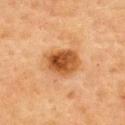biopsy_status: not biopsied; imaged during a skin examination
lesion_size:
  long_diameter_mm_approx: 4.5
automated_metrics:
  cielab_L: 47
  cielab_a: 24
  cielab_b: 39
  vs_skin_darker_L: 14.0
  nevus_likeness_0_100: 95
  lesion_detection_confidence_0_100: 100
site: upper back
image:
  source: total-body photography crop
  field_of_view_mm: 15
patient:
  sex: female
  age_approx: 60